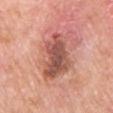<record>
<biopsy_status>not biopsied; imaged during a skin examination</biopsy_status>
<patient>
  <sex>male</sex>
  <age_approx>80</age_approx>
</patient>
<site>chest</site>
<lighting>white-light</lighting>
<automated_metrics>
  <nevus_likeness_0_100>0</nevus_likeness_0_100>
</automated_metrics>
<lesion_size>
  <long_diameter_mm_approx>6.0</long_diameter_mm_approx>
</lesion_size>
<image>
  <source>total-body photography crop</source>
  <field_of_view_mm>15</field_of_view_mm>
</image>
</record>